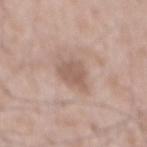Q: Is there a histopathology result?
A: no biopsy performed (imaged during a skin exam)
Q: Patient demographics?
A: male, approximately 50 years of age
Q: How was this image acquired?
A: ~15 mm tile from a whole-body skin photo
Q: How was the tile lit?
A: white-light
Q: Where on the body is the lesion?
A: the mid back
Q: Lesion size?
A: ~4 mm (longest diameter)